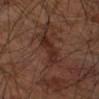follow-up: catalogued during a skin exam; not biopsied | automated lesion analysis: an average lesion color of about L≈26 a*≈19 b*≈23 (CIELAB), about 7 CIELAB-L* units darker than the surrounding skin, and a normalized border contrast of about 8; a border-irregularity rating of about 4.5/10, internal color variation of about 2.5 on a 0–10 scale, and radial color variation of about 0.5; a classifier nevus-likeness of about 20/100 and lesion-presence confidence of about 95/100 | body site: the arm | image: ~15 mm crop, total-body skin-cancer survey | illumination: cross-polarized | subject: male, aged 63–67.